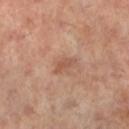The lesion was tiled from a total-body skin photograph and was not biopsied.
On the left lower leg.
Automated tile analysis of the lesion measured an outline eccentricity of about 0.9 (0 = round, 1 = elongated) and a shape-asymmetry score of about 0.4 (0 = symmetric). The software also gave a mean CIELAB color near L≈55 a*≈23 b*≈30, roughly 8 lightness units darker than nearby skin, and a normalized border contrast of about 6. The software also gave a border-irregularity index near 4/10, a within-lesion color-variation index near 2/10, and peripheral color asymmetry of about 0.5. It also reported a lesion-detection confidence of about 100/100.
A female patient, in their 60s.
A close-up tile cropped from a whole-body skin photograph, about 15 mm across.
This is a cross-polarized tile.
Longest diameter approximately 3 mm.